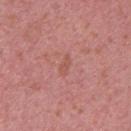Assessment:
The lesion was tiled from a total-body skin photograph and was not biopsied.
Background:
On the upper back. The subject is a female in their 40s. Captured under white-light illumination. A 15 mm crop from a total-body photograph taken for skin-cancer surveillance.